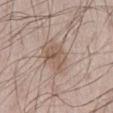Findings:
• biopsy status · catalogued during a skin exam; not biopsied
• imaging modality · 15 mm crop, total-body photography
• patient · male, approximately 65 years of age
• body site · the abdomen
• lesion size · ≈3.5 mm